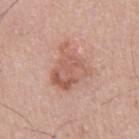Q: Was this lesion biopsied?
A: catalogued during a skin exam; not biopsied
Q: What is the lesion's diameter?
A: about 5.5 mm
Q: What kind of image is this?
A: ~15 mm crop, total-body skin-cancer survey
Q: Illumination type?
A: white-light illumination
Q: Patient demographics?
A: male, in their mid-60s
Q: Lesion location?
A: the right upper arm